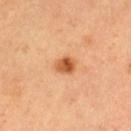notes: catalogued during a skin exam; not biopsied | subject: male, aged around 50 | diameter: ~2.5 mm (longest diameter) | tile lighting: cross-polarized illumination | body site: the left thigh | image: ~15 mm crop, total-body skin-cancer survey | automated metrics: a footprint of about 4.5 mm², a shape eccentricity near 0.65, and a symmetry-axis asymmetry near 0.2; an average lesion color of about L≈57 a*≈28 b*≈43 (CIELAB) and roughly 14 lightness units darker than nearby skin; a nevus-likeness score of about 95/100.